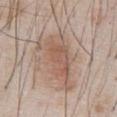Assessment:
Captured during whole-body skin photography for melanoma surveillance; the lesion was not biopsied.
Acquisition and patient details:
A male subject in their mid-50s. A 15 mm crop from a total-body photograph taken for skin-cancer surveillance. The lesion is located on the chest. Automated tile analysis of the lesion measured a lesion area of about 18 mm², an outline eccentricity of about 0.9 (0 = round, 1 = elongated), and a shape-asymmetry score of about 0.35 (0 = symmetric). The software also gave about 9 CIELAB-L* units darker than the surrounding skin and a normalized lesion–skin contrast near 6.5. The lesion's longest dimension is about 8 mm.